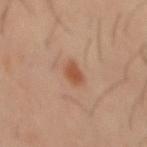No biopsy was performed on this lesion — it was imaged during a full skin examination and was not determined to be concerning. From the mid back. The recorded lesion diameter is about 3 mm. A male patient, aged 38 to 42. Cropped from a total-body skin-imaging series; the visible field is about 15 mm. Imaged with cross-polarized lighting.